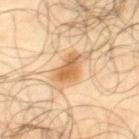The lesion was tiled from a total-body skin photograph and was not biopsied. A 15 mm close-up extracted from a 3D total-body photography capture. The subject is a male aged around 65. From the right thigh. The tile uses cross-polarized illumination. About 6 mm across.